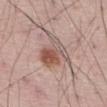workup: imaged on a skin check; not biopsied | imaging modality: total-body-photography crop, ~15 mm field of view | subject: male, aged around 60 | size: ~4.5 mm (longest diameter) | tile lighting: white-light illumination | body site: the abdomen | automated lesion analysis: a footprint of about 13 mm² and a shape eccentricity near 0.55; a lesion color around L≈55 a*≈19 b*≈24 in CIELAB, about 10 CIELAB-L* units darker than the surrounding skin, and a normalized border contrast of about 7; a nevus-likeness score of about 95/100 and a lesion-detection confidence of about 100/100.